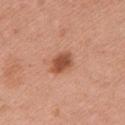Clinical impression: Captured during whole-body skin photography for melanoma surveillance; the lesion was not biopsied. Clinical summary: A 15 mm close-up tile from a total-body photography series done for melanoma screening. The lesion is located on the left upper arm. The tile uses white-light illumination. The patient is a female roughly 50 years of age. The total-body-photography lesion software estimated a lesion area of about 5.5 mm², an eccentricity of roughly 0.7, and two-axis asymmetry of about 0.2. And it measured a lesion color around L≈51 a*≈27 b*≈33 in CIELAB, a lesion–skin lightness drop of about 14, and a normalized border contrast of about 9. It also reported a border-irregularity rating of about 2/10, internal color variation of about 3.5 on a 0–10 scale, and peripheral color asymmetry of about 1.